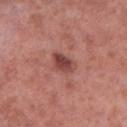Q: Is there a histopathology result?
A: no biopsy performed (imaged during a skin exam)
Q: Where on the body is the lesion?
A: the right lower leg
Q: What kind of image is this?
A: ~15 mm tile from a whole-body skin photo
Q: What lighting was used for the tile?
A: white-light
Q: What is the lesion's diameter?
A: ~3 mm (longest diameter)
Q: What did automated image analysis measure?
A: a footprint of about 5 mm², an eccentricity of roughly 0.75, and two-axis asymmetry of about 0.25; an automated nevus-likeness rating near 45 out of 100 and a detector confidence of about 100 out of 100 that the crop contains a lesion
Q: Patient demographics?
A: male, approximately 55 years of age